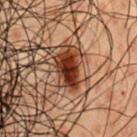workup: catalogued during a skin exam; not biopsied | image-analysis metrics: an area of roughly 8 mm², an eccentricity of roughly 0.8, and a symmetry-axis asymmetry near 0.3; a border-irregularity rating of about 3/10 and a color-variation rating of about 4.5/10 | lighting: cross-polarized illumination | body site: the chest | image: ~15 mm tile from a whole-body skin photo | patient: male, aged around 50.